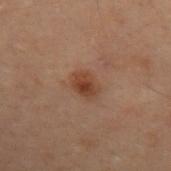No biopsy was performed on this lesion — it was imaged during a full skin examination and was not determined to be concerning. The recorded lesion diameter is about 3 mm. Cropped from a total-body skin-imaging series; the visible field is about 15 mm. Automated image analysis of the tile measured an area of roughly 5 mm², an eccentricity of roughly 0.65, and two-axis asymmetry of about 0.2. The software also gave border irregularity of about 2 on a 0–10 scale, internal color variation of about 3.5 on a 0–10 scale, and radial color variation of about 1. On the left forearm. The subject is a male in their 50s. Imaged with cross-polarized lighting.